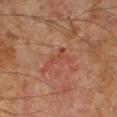Acquisition and patient details: Imaged with cross-polarized lighting. Automated image analysis of the tile measured border irregularity of about 9.5 on a 0–10 scale and a peripheral color-asymmetry measure near 0. The software also gave a classifier nevus-likeness of about 0/100 and a lesion-detection confidence of about 95/100. A male patient, aged 58–62. The lesion's longest dimension is about 4 mm. The lesion is on the right lower leg. Cropped from a whole-body photographic skin survey; the tile spans about 15 mm.